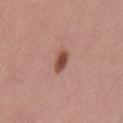Located on the lower back. A female patient in their mid- to late 20s. A 15 mm close-up tile from a total-body photography series done for melanoma screening. The tile uses white-light illumination. The recorded lesion diameter is about 3 mm.